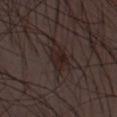imaging modality = total-body-photography crop, ~15 mm field of view | patient = male, approximately 50 years of age | lesion diameter = ~3.5 mm (longest diameter) | image-analysis metrics = a footprint of about 5.5 mm², a shape eccentricity near 0.7, and a symmetry-axis asymmetry near 0.3; peripheral color asymmetry of about 1.